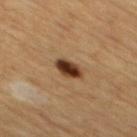Findings:
* notes: catalogued during a skin exam; not biopsied
* anatomic site: the mid back
* lesion diameter: ≈3 mm
* lighting: cross-polarized illumination
* image: total-body-photography crop, ~15 mm field of view
* subject: male, aged 58–62
* automated metrics: a lesion area of about 5.5 mm²